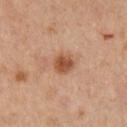  biopsy_status: not biopsied; imaged during a skin examination
  image:
    source: total-body photography crop
    field_of_view_mm: 15
  lighting: cross-polarized
  site: right upper arm
  patient:
    sex: female
    age_approx: 45
  automated_metrics:
    cielab_L: 50
    cielab_a: 23
    cielab_b: 33
    vs_skin_darker_L: 11.0
    vs_skin_contrast_norm: 8.5
    border_irregularity_0_10: 2.0
    color_variation_0_10: 3.5
    peripheral_color_asymmetry: 1.5